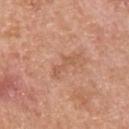{
  "biopsy_status": "not biopsied; imaged during a skin examination",
  "image": {
    "source": "total-body photography crop",
    "field_of_view_mm": 15
  },
  "patient": {
    "sex": "male",
    "age_approx": 60
  },
  "lighting": "white-light",
  "site": "back",
  "automated_metrics": {
    "nevus_likeness_0_100": 0,
    "lesion_detection_confidence_0_100": 100
  },
  "lesion_size": {
    "long_diameter_mm_approx": 3.5
  }
}